Captured under white-light illumination; approximately 7.5 mm at its widest; the lesion is located on the front of the torso; a roughly 15 mm field-of-view crop from a total-body skin photograph; An algorithmic analysis of the crop reported an automated nevus-likeness rating near 40 out of 100; the patient is a male aged 53–57:
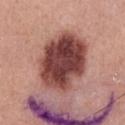histopathologic diagnosis: a dysplastic (Clark) nevus.